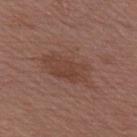Part of a total-body skin-imaging series; this lesion was reviewed on a skin check and was not flagged for biopsy. Longest diameter approximately 7.5 mm. A 15 mm crop from a total-body photograph taken for skin-cancer surveillance. A female subject, aged 53–57. Located on the right upper arm. The lesion-visualizer software estimated a mean CIELAB color near L≈43 a*≈20 b*≈25, a lesion–skin lightness drop of about 6, and a normalized border contrast of about 5.5. The analysis additionally found border irregularity of about 4.5 on a 0–10 scale. This is a white-light tile.